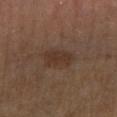  biopsy_status: not biopsied; imaged during a skin examination
  automated_metrics:
    cielab_L: 31
    cielab_a: 15
    cielab_b: 24
    vs_skin_darker_L: 6.0
    vs_skin_contrast_norm: 6.5
    color_variation_0_10: 2.0
    peripheral_color_asymmetry: 1.0
    nevus_likeness_0_100: 10
    lesion_detection_confidence_0_100: 100
  patient:
    sex: male
    age_approx: 65
  site: leg
  lighting: cross-polarized
  lesion_size:
    long_diameter_mm_approx: 3.5
  image:
    source: total-body photography crop
    field_of_view_mm: 15